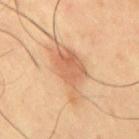Clinical impression: Imaged during a routine full-body skin examination; the lesion was not biopsied and no histopathology is available. Context: Located on the mid back. A roughly 15 mm field-of-view crop from a total-body skin photograph. The total-body-photography lesion software estimated a border-irregularity index near 5/10, internal color variation of about 4 on a 0–10 scale, and radial color variation of about 1.5. Imaged with cross-polarized lighting. A male patient about 65 years old.